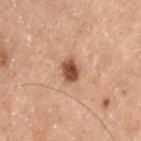Imaged during a routine full-body skin examination; the lesion was not biopsied and no histopathology is available. Located on the left thigh. Cropped from a total-body skin-imaging series; the visible field is about 15 mm. The recorded lesion diameter is about 2.5 mm. The lesion-visualizer software estimated a lesion area of about 5.5 mm², a shape eccentricity near 0.5, and two-axis asymmetry of about 0.2. And it measured an average lesion color of about L≈38 a*≈18 b*≈25 (CIELAB) and about 12 CIELAB-L* units darker than the surrounding skin. The patient is a male aged 68–72.